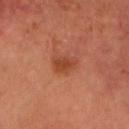This lesion was catalogued during total-body skin photography and was not selected for biopsy.
A male subject, about 50 years old.
The recorded lesion diameter is about 3 mm.
Cropped from a total-body skin-imaging series; the visible field is about 15 mm.
The total-body-photography lesion software estimated a nevus-likeness score of about 70/100.
Captured under cross-polarized illumination.
Located on the head or neck.